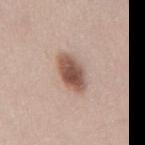{
  "biopsy_status": "not biopsied; imaged during a skin examination",
  "automated_metrics": {
    "area_mm2_approx": 9.5,
    "shape_asymmetry": 0.15,
    "border_irregularity_0_10": 1.5,
    "color_variation_0_10": 5.0,
    "peripheral_color_asymmetry": 1.5,
    "nevus_likeness_0_100": 100,
    "lesion_detection_confidence_0_100": 100
  },
  "patient": {
    "sex": "male",
    "age_approx": 45
  },
  "lesion_size": {
    "long_diameter_mm_approx": 4.5
  },
  "image": {
    "source": "total-body photography crop",
    "field_of_view_mm": 15
  },
  "site": "back"
}